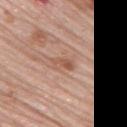biopsy status: total-body-photography surveillance lesion; no biopsy | location: the right upper arm | image source: 15 mm crop, total-body photography | lesion size: about 2.5 mm | tile lighting: white-light | patient: female, in their mid-60s.